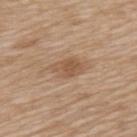Part of a total-body skin-imaging series; this lesion was reviewed on a skin check and was not flagged for biopsy.
About 3.5 mm across.
Cropped from a total-body skin-imaging series; the visible field is about 15 mm.
The lesion is located on the upper back.
A female subject, aged approximately 75.